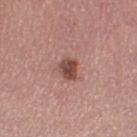biopsy status=imaged on a skin check; not biopsied
size=about 2.5 mm
imaging modality=15 mm crop, total-body photography
patient=female, in their mid- to late 30s
location=the leg